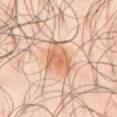Q: Was a biopsy performed?
A: catalogued during a skin exam; not biopsied
Q: Automated lesion metrics?
A: a lesion area of about 12 mm², an eccentricity of roughly 0.65, and a symmetry-axis asymmetry near 0.3; internal color variation of about 5 on a 0–10 scale and radial color variation of about 1.5
Q: Lesion location?
A: the front of the torso
Q: Patient demographics?
A: male, aged 63–67
Q: Lesion size?
A: about 4.5 mm
Q: How was this image acquired?
A: total-body-photography crop, ~15 mm field of view
Q: How was the tile lit?
A: cross-polarized